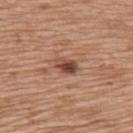{
  "biopsy_status": "not biopsied; imaged during a skin examination",
  "lighting": "white-light",
  "patient": {
    "sex": "female",
    "age_approx": 75
  },
  "image": {
    "source": "total-body photography crop",
    "field_of_view_mm": 15
  },
  "lesion_size": {
    "long_diameter_mm_approx": 3.0
  },
  "site": "upper back"
}